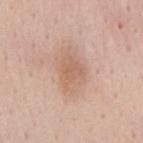Clinical impression:
This lesion was catalogued during total-body skin photography and was not selected for biopsy.
Background:
On the mid back. A lesion tile, about 15 mm wide, cut from a 3D total-body photograph. This is a white-light tile. A male subject aged around 55. Approximately 4 mm at its widest. The total-body-photography lesion software estimated a lesion area of about 7 mm², a shape eccentricity near 0.65, and two-axis asymmetry of about 0.4. The software also gave an automated nevus-likeness rating near 15 out of 100 and lesion-presence confidence of about 100/100.